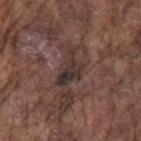Recorded during total-body skin imaging; not selected for excision or biopsy.
From the left upper arm.
A close-up tile cropped from a whole-body skin photograph, about 15 mm across.
Captured under white-light illumination.
The patient is a male approximately 75 years of age.
Measured at roughly 4.5 mm in maximum diameter.